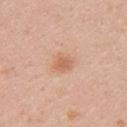The lesion was tiled from a total-body skin photograph and was not biopsied. This is a white-light tile. Approximately 3 mm at its widest. On the upper back. A 15 mm crop from a total-body photograph taken for skin-cancer surveillance. A female patient, aged approximately 20.